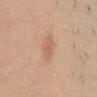Background:
This is a white-light tile. A 15 mm close-up tile from a total-body photography series done for melanoma screening. The lesion's longest dimension is about 3 mm. Located on the left upper arm. A female patient approximately 30 years of age. Automated tile analysis of the lesion measured a lesion area of about 5 mm² and two-axis asymmetry of about 0.25. And it measured roughly 7 lightness units darker than nearby skin and a normalized border contrast of about 5.